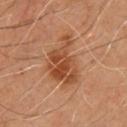{
  "lesion_size": {
    "long_diameter_mm_approx": 6.0
  },
  "site": "chest",
  "automated_metrics": {
    "vs_skin_darker_L": 10.0,
    "vs_skin_contrast_norm": 8.0,
    "border_irregularity_0_10": 6.0,
    "color_variation_0_10": 5.0,
    "peripheral_color_asymmetry": 1.5
  },
  "lighting": "cross-polarized",
  "image": {
    "source": "total-body photography crop",
    "field_of_view_mm": 15
  },
  "patient": {
    "sex": "male",
    "age_approx": 55
  }
}